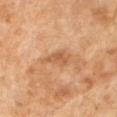follow-up: no biopsy performed (imaged during a skin exam); lesion diameter: about 3 mm; subject: male, aged around 65; image: ~15 mm tile from a whole-body skin photo; automated lesion analysis: a lesion area of about 4.5 mm², a shape eccentricity near 0.75, and two-axis asymmetry of about 0.35; lighting: cross-polarized illumination.